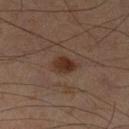Q: Is there a histopathology result?
A: no biopsy performed (imaged during a skin exam)
Q: Where on the body is the lesion?
A: the right lower leg
Q: What kind of image is this?
A: total-body-photography crop, ~15 mm field of view
Q: Who is the patient?
A: male, aged approximately 60